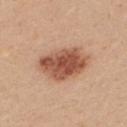workup: no biopsy performed (imaged during a skin exam); lesion diameter: ≈6 mm; location: the upper back; image: 15 mm crop, total-body photography; subject: female, in their mid- to late 20s; illumination: white-light illumination.